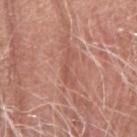The lesion was tiled from a total-body skin photograph and was not biopsied. Measured at roughly 4 mm in maximum diameter. A male subject, aged 78 to 82. The lesion is located on the right forearm. Cropped from a total-body skin-imaging series; the visible field is about 15 mm.